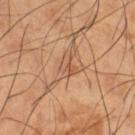The lesion was photographed on a routine skin check and not biopsied; there is no pathology result. Located on the leg. Longest diameter approximately 4 mm. The tile uses cross-polarized illumination. A roughly 15 mm field-of-view crop from a total-body skin photograph. A male patient, in their mid-60s. Automated tile analysis of the lesion measured a footprint of about 5 mm², an eccentricity of roughly 0.85, and two-axis asymmetry of about 0.65. And it measured a peripheral color-asymmetry measure near 1.5.